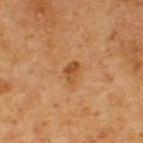Recorded during total-body skin imaging; not selected for excision or biopsy.
A male patient, aged approximately 75.
The lesion is located on the upper back.
Cropped from a total-body skin-imaging series; the visible field is about 15 mm.
Imaged with cross-polarized lighting.
The lesion's longest dimension is about 2.5 mm.
Automated image analysis of the tile measured a footprint of about 3.5 mm², an eccentricity of roughly 0.8, and two-axis asymmetry of about 0.3. The analysis additionally found a lesion color around L≈39 a*≈20 b*≈34 in CIELAB, a lesion–skin lightness drop of about 8, and a normalized lesion–skin contrast near 7.